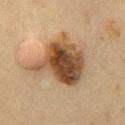Recorded during total-body skin imaging; not selected for excision or biopsy.
From the chest.
The lesion's longest dimension is about 6.5 mm.
This is a cross-polarized tile.
Cropped from a whole-body photographic skin survey; the tile spans about 15 mm.
The patient is a male approximately 60 years of age.
Automated tile analysis of the lesion measured an area of roughly 30 mm², an eccentricity of roughly 0.3, and a shape-asymmetry score of about 0.4 (0 = symmetric). The analysis additionally found a lesion color around L≈41 a*≈15 b*≈29 in CIELAB, about 14 CIELAB-L* units darker than the surrounding skin, and a normalized border contrast of about 11. And it measured a nevus-likeness score of about 35/100 and lesion-presence confidence of about 100/100.